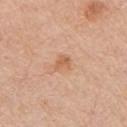Q: What did automated image analysis measure?
A: an area of roughly 3 mm² and an eccentricity of roughly 0.6; internal color variation of about 1 on a 0–10 scale and peripheral color asymmetry of about 0.5
Q: Where on the body is the lesion?
A: the left upper arm
Q: What kind of image is this?
A: 15 mm crop, total-body photography
Q: Who is the patient?
A: female, in their mid-60s
Q: How was the tile lit?
A: white-light illumination